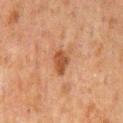Impression:
Captured during whole-body skin photography for melanoma surveillance; the lesion was not biopsied.
Acquisition and patient details:
The lesion is located on the back. The subject is a male aged 58 to 62. The tile uses cross-polarized illumination. A region of skin cropped from a whole-body photographic capture, roughly 15 mm wide. Measured at roughly 3 mm in maximum diameter.